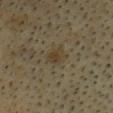This lesion was catalogued during total-body skin photography and was not selected for biopsy. The lesion is located on the head or neck. A close-up tile cropped from a whole-body skin photograph, about 15 mm across. The tile uses cross-polarized illumination. A male patient, about 60 years old.